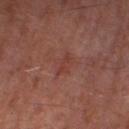A male patient, aged around 65. On the right thigh. Imaged with cross-polarized lighting. Measured at roughly 2.5 mm in maximum diameter. A lesion tile, about 15 mm wide, cut from a 3D total-body photograph.